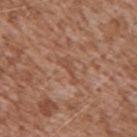Q: Was this lesion biopsied?
A: no biopsy performed (imaged during a skin exam)
Q: Patient demographics?
A: male, approximately 45 years of age
Q: What is the imaging modality?
A: ~15 mm tile from a whole-body skin photo
Q: Where on the body is the lesion?
A: the right upper arm
Q: How large is the lesion?
A: ~3.5 mm (longest diameter)
Q: Illumination type?
A: white-light illumination